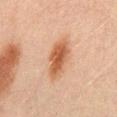The lesion was tiled from a total-body skin photograph and was not biopsied.
On the mid back.
A 15 mm crop from a total-body photograph taken for skin-cancer surveillance.
A male subject, about 45 years old.
The tile uses cross-polarized illumination.
The lesion-visualizer software estimated a lesion color around L≈47 a*≈20 b*≈31 in CIELAB and a normalized lesion–skin contrast near 9. The analysis additionally found a color-variation rating of about 4.5/10 and a peripheral color-asymmetry measure near 1.5. The software also gave a nevus-likeness score of about 100/100 and a detector confidence of about 100 out of 100 that the crop contains a lesion.
About 5 mm across.